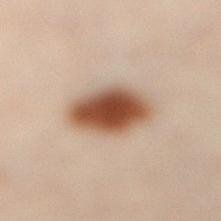Clinical impression: Imaged during a routine full-body skin examination; the lesion was not biopsied and no histopathology is available. Context: A male patient about 50 years old. A close-up tile cropped from a whole-body skin photograph, about 15 mm across. Located on the leg. The tile uses cross-polarized illumination. The lesion-visualizer software estimated a shape eccentricity near 0.7 and two-axis asymmetry of about 0.1. About 5 mm across.